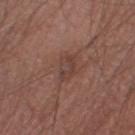Impression: Part of a total-body skin-imaging series; this lesion was reviewed on a skin check and was not flagged for biopsy. Acquisition and patient details: On the leg. A male subject roughly 55 years of age. An algorithmic analysis of the crop reported a border-irregularity index near 2/10 and peripheral color asymmetry of about 1. This is a white-light tile. A close-up tile cropped from a whole-body skin photograph, about 15 mm across.